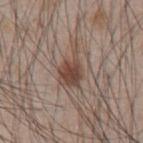Clinical summary:
A 15 mm close-up tile from a total-body photography series done for melanoma screening. This is a white-light tile. The patient is a male in their mid- to late 40s. Located on the front of the torso.